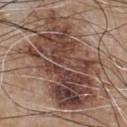Clinical summary: On the chest. The total-body-photography lesion software estimated a lesion area of about 65 mm². It also reported a mean CIELAB color near L≈44 a*≈18 b*≈24 and about 14 CIELAB-L* units darker than the surrounding skin. The software also gave a border-irregularity rating of about 5/10, internal color variation of about 9 on a 0–10 scale, and peripheral color asymmetry of about 3.5. And it measured a classifier nevus-likeness of about 5/100. A 15 mm close-up extracted from a 3D total-body photography capture. A male subject about 65 years old. Approximately 11.5 mm at its widest.